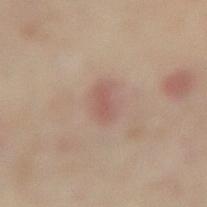The tile uses cross-polarized illumination. Approximately 2.5 mm at its widest. The subject is a female aged around 35. Cropped from a whole-body photographic skin survey; the tile spans about 15 mm. From the right lower leg.